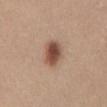{"biopsy_status": "not biopsied; imaged during a skin examination", "image": {"source": "total-body photography crop", "field_of_view_mm": 15}, "site": "front of the torso", "patient": {"sex": "female", "age_approx": 30}}